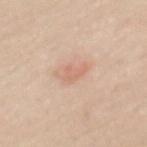Clinical impression: Captured during whole-body skin photography for melanoma surveillance; the lesion was not biopsied. Clinical summary: On the mid back. A 15 mm crop from a total-body photograph taken for skin-cancer surveillance. A female subject, in their mid- to late 40s.